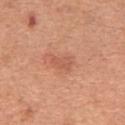tile lighting = white-light; image source = ~15 mm crop, total-body skin-cancer survey; size = about 2.5 mm; body site = the abdomen; patient = male, aged 63–67.